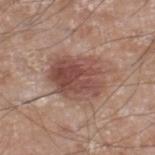notes — no biopsy performed (imaged during a skin exam) | patient — male, roughly 60 years of age | automated lesion analysis — an area of roughly 21 mm² and an eccentricity of roughly 0.65; a nevus-likeness score of about 80/100 and lesion-presence confidence of about 100/100 | acquisition — 15 mm crop, total-body photography | site — the right lower leg | lesion diameter — about 6 mm.